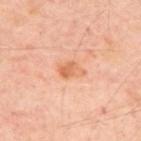follow-up — catalogued during a skin exam; not biopsied | acquisition — ~15 mm crop, total-body skin-cancer survey | patient — aged approximately 55 | site — the upper back.